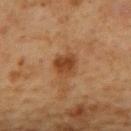Image and clinical context:
The subject is a male about 60 years old. Automated image analysis of the tile measured a footprint of about 6.5 mm², an eccentricity of roughly 0.45, and a symmetry-axis asymmetry near 0.25. The analysis additionally found a normalized border contrast of about 8. The analysis additionally found a color-variation rating of about 4/10 and radial color variation of about 1.5. Captured under cross-polarized illumination. The lesion is on the back. Longest diameter approximately 3 mm. Cropped from a total-body skin-imaging series; the visible field is about 15 mm.